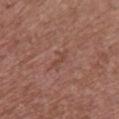Image and clinical context: Automated tile analysis of the lesion measured a border-irregularity index near 4/10, a within-lesion color-variation index near 0/10, and peripheral color asymmetry of about 0. On the front of the torso. The tile uses white-light illumination. A female patient aged approximately 65. A 15 mm crop from a total-body photograph taken for skin-cancer surveillance.